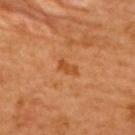Case summary:
– biopsy status · total-body-photography surveillance lesion; no biopsy
– illumination · cross-polarized
– patient · female, aged around 45
– body site · the upper back
– diameter · about 2.5 mm
– image source · ~15 mm crop, total-body skin-cancer survey